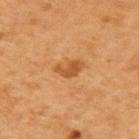Impression:
Part of a total-body skin-imaging series; this lesion was reviewed on a skin check and was not flagged for biopsy.
Clinical summary:
Longest diameter approximately 3 mm. Located on the right upper arm. This image is a 15 mm lesion crop taken from a total-body photograph. Captured under cross-polarized illumination. A male patient aged 53 to 57.